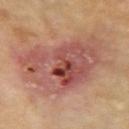Part of a total-body skin-imaging series; this lesion was reviewed on a skin check and was not flagged for biopsy. The lesion's longest dimension is about 10.5 mm. The lesion-visualizer software estimated an area of roughly 50 mm², an eccentricity of roughly 0.8, and a symmetry-axis asymmetry near 0.25. The analysis additionally found a lesion color around L≈52 a*≈24 b*≈26 in CIELAB and a lesion-to-skin contrast of about 8.5 (normalized; higher = more distinct). And it measured a classifier nevus-likeness of about 0/100 and a detector confidence of about 100 out of 100 that the crop contains a lesion. Imaged with cross-polarized lighting. Cropped from a whole-body photographic skin survey; the tile spans about 15 mm. The subject is a male aged around 70. From the right upper arm.